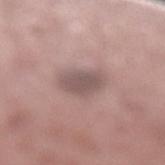This lesion was catalogued during total-body skin photography and was not selected for biopsy.
Imaged with white-light lighting.
Approximately 4 mm at its widest.
A region of skin cropped from a whole-body photographic capture, roughly 15 mm wide.
The total-body-photography lesion software estimated a lesion area of about 8 mm² and an outline eccentricity of about 0.7 (0 = round, 1 = elongated). The software also gave a lesion color around L≈53 a*≈16 b*≈18 in CIELAB, about 10 CIELAB-L* units darker than the surrounding skin, and a normalized border contrast of about 7. It also reported a classifier nevus-likeness of about 0/100 and lesion-presence confidence of about 95/100.
A male patient, approximately 55 years of age.
Located on the left lower leg.